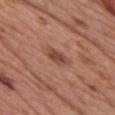The lesion was photographed on a routine skin check and not biopsied; there is no pathology result.
From the chest.
A female patient approximately 60 years of age.
A region of skin cropped from a whole-body photographic capture, roughly 15 mm wide.
The tile uses white-light illumination.